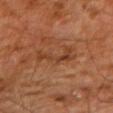workup = catalogued during a skin exam; not biopsied
patient = male, aged 58–62
lighting = cross-polarized
acquisition = ~15 mm crop, total-body skin-cancer survey
lesion size = ~4 mm (longest diameter)
site = the right upper arm